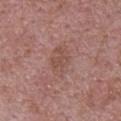{"biopsy_status": "not biopsied; imaged during a skin examination", "patient": {"sex": "male", "age_approx": 70}, "lighting": "white-light", "lesion_size": {"long_diameter_mm_approx": 4.0}, "site": "back", "image": {"source": "total-body photography crop", "field_of_view_mm": 15}}